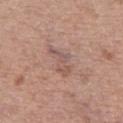Clinical impression:
The lesion was tiled from a total-body skin photograph and was not biopsied.
Acquisition and patient details:
Imaged with white-light lighting. A female subject aged 58 to 62. Located on the right thigh. The recorded lesion diameter is about 4 mm. A region of skin cropped from a whole-body photographic capture, roughly 15 mm wide.